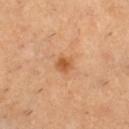| feature | finding |
|---|---|
| tile lighting | cross-polarized |
| image | 15 mm crop, total-body photography |
| lesion diameter | ~2 mm (longest diameter) |
| location | the leg |
| image-analysis metrics | a lesion area of about 3 mm² and a symmetry-axis asymmetry near 0.15; a classifier nevus-likeness of about 80/100 and a lesion-detection confidence of about 100/100 |
| subject | female, in their 30s |